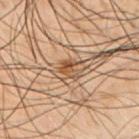Context: Captured under cross-polarized illumination. The subject is a male aged around 50. The lesion is located on the back. A 15 mm crop from a total-body photograph taken for skin-cancer surveillance.